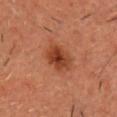Imaged during a routine full-body skin examination; the lesion was not biopsied and no histopathology is available.
A male subject aged 58 to 62.
The tile uses cross-polarized illumination.
The recorded lesion diameter is about 4 mm.
The lesion is located on the head or neck.
A 15 mm crop from a total-body photograph taken for skin-cancer surveillance.